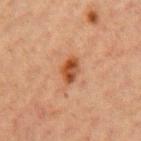| key | value |
|---|---|
| follow-up | catalogued during a skin exam; not biopsied |
| patient | female, approximately 40 years of age |
| body site | the left upper arm |
| acquisition | ~15 mm tile from a whole-body skin photo |